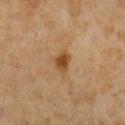Captured during whole-body skin photography for melanoma surveillance; the lesion was not biopsied.
Located on the chest.
The tile uses cross-polarized illumination.
Cropped from a whole-body photographic skin survey; the tile spans about 15 mm.
The total-body-photography lesion software estimated an average lesion color of about L≈39 a*≈17 b*≈32 (CIELAB), a lesion–skin lightness drop of about 9, and a normalized border contrast of about 8.5.
A male patient, aged approximately 60.
About 3 mm across.